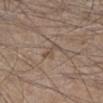biopsy status: no biopsy performed (imaged during a skin exam) | acquisition: ~15 mm crop, total-body skin-cancer survey | location: the leg | illumination: white-light | automated metrics: a shape eccentricity near 0.9 and a symmetry-axis asymmetry near 0.45; a mean CIELAB color near L≈49 a*≈14 b*≈25, a lesion–skin lightness drop of about 7, and a normalized lesion–skin contrast near 5.5; a border-irregularity rating of about 5/10, internal color variation of about 0 on a 0–10 scale, and radial color variation of about 0 | subject: male, in their mid- to late 40s | lesion diameter: ~3 mm (longest diameter).